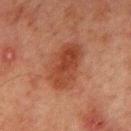notes: catalogued during a skin exam; not biopsied | subject: male, aged approximately 65 | body site: the chest | imaging modality: ~15 mm crop, total-body skin-cancer survey | tile lighting: cross-polarized | lesion diameter: about 6 mm.